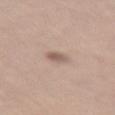Part of a total-body skin-imaging series; this lesion was reviewed on a skin check and was not flagged for biopsy.
On the right thigh.
The total-body-photography lesion software estimated a border-irregularity rating of about 1.5/10, a color-variation rating of about 4/10, and a peripheral color-asymmetry measure near 1.5.
The subject is a female aged around 55.
About 3 mm across.
Cropped from a whole-body photographic skin survey; the tile spans about 15 mm.